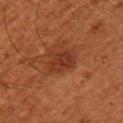  automated_metrics:
    area_mm2_approx: 7.5
    eccentricity: 0.55
    shape_asymmetry: 0.2
    cielab_L: 33
    cielab_a: 26
    cielab_b: 31
    vs_skin_darker_L: 7.0
    vs_skin_contrast_norm: 7.0
    lesion_detection_confidence_0_100: 100
  lighting: cross-polarized
  site: left upper arm
  image:
    source: total-body photography crop
    field_of_view_mm: 15
  patient:
    sex: male
    age_approx: 45
  lesion_size:
    long_diameter_mm_approx: 3.5